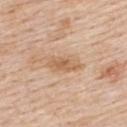Q: Was this lesion biopsied?
A: no biopsy performed (imaged during a skin exam)
Q: Lesion size?
A: ~4.5 mm (longest diameter)
Q: Automated lesion metrics?
A: a within-lesion color-variation index near 3.5/10 and radial color variation of about 1; an automated nevus-likeness rating near 0 out of 100 and a detector confidence of about 100 out of 100 that the crop contains a lesion
Q: Who is the patient?
A: female, about 70 years old
Q: What lighting was used for the tile?
A: white-light
Q: How was this image acquired?
A: total-body-photography crop, ~15 mm field of view
Q: Where on the body is the lesion?
A: the upper back